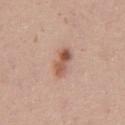This lesion was catalogued during total-body skin photography and was not selected for biopsy. Longest diameter approximately 4 mm. Imaged with white-light lighting. A male subject in their 40s. A 15 mm crop from a total-body photograph taken for skin-cancer surveillance. Located on the front of the torso.